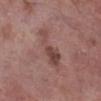Clinical summary: Imaged with white-light lighting. A close-up tile cropped from a whole-body skin photograph, about 15 mm across. The patient is a male aged approximately 70. Automated tile analysis of the lesion measured a color-variation rating of about 3/10. The lesion is located on the right lower leg.